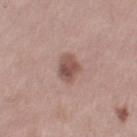Captured during whole-body skin photography for melanoma surveillance; the lesion was not biopsied. A lesion tile, about 15 mm wide, cut from a 3D total-body photograph. The lesion-visualizer software estimated a lesion area of about 6 mm² and a shape-asymmetry score of about 0.2 (0 = symmetric). The analysis additionally found a lesion color around L≈51 a*≈20 b*≈24 in CIELAB and a normalized border contrast of about 8. The software also gave internal color variation of about 4.5 on a 0–10 scale and a peripheral color-asymmetry measure near 1.5. The analysis additionally found a nevus-likeness score of about 70/100 and a detector confidence of about 100 out of 100 that the crop contains a lesion. Located on the arm. The lesion's longest dimension is about 3 mm. A female patient, about 40 years old.